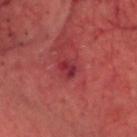• biopsy status — catalogued during a skin exam; not biopsied
• tile lighting — cross-polarized illumination
• subject — male, in their mid- to late 60s
• TBP lesion metrics — an average lesion color of about L≈34 a*≈36 b*≈21 (CIELAB), a lesion–skin lightness drop of about 8, and a normalized lesion–skin contrast near 7; a within-lesion color-variation index near 3.5/10
• body site — the head or neck
• image — ~15 mm tile from a whole-body skin photo